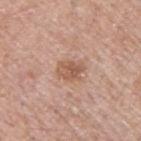An algorithmic analysis of the crop reported a footprint of about 6 mm², a shape eccentricity near 0.65, and two-axis asymmetry of about 0.2. The analysis additionally found an automated nevus-likeness rating near 15 out of 100 and a lesion-detection confidence of about 100/100. The lesion is on the back. Measured at roughly 3 mm in maximum diameter. Captured under white-light illumination. Cropped from a total-body skin-imaging series; the visible field is about 15 mm. A male patient, in their mid- to late 50s.